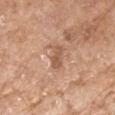Q: How was this image acquired?
A: 15 mm crop, total-body photography
Q: Who is the patient?
A: female, aged 73–77
Q: Automated lesion metrics?
A: an average lesion color of about L≈56 a*≈21 b*≈31 (CIELAB), a lesion–skin lightness drop of about 9, and a normalized border contrast of about 6.5; a border-irregularity rating of about 3/10 and radial color variation of about 1
Q: What is the anatomic site?
A: the right forearm